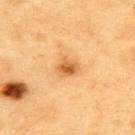Q: Was this lesion biopsied?
A: no biopsy performed (imaged during a skin exam)
Q: Where on the body is the lesion?
A: the upper back
Q: What lighting was used for the tile?
A: cross-polarized illumination
Q: What are the patient's age and sex?
A: male, aged around 60
Q: What kind of image is this?
A: 15 mm crop, total-body photography
Q: Automated lesion metrics?
A: a border-irregularity index near 2.5/10 and radial color variation of about 1.5; an automated nevus-likeness rating near 80 out of 100